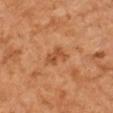| key | value |
|---|---|
| workup | total-body-photography surveillance lesion; no biopsy |
| anatomic site | the right arm |
| image source | 15 mm crop, total-body photography |
| illumination | cross-polarized illumination |
| diameter | about 3.5 mm |
| subject | male, roughly 60 years of age |